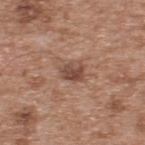workup — total-body-photography surveillance lesion; no biopsy | image — ~15 mm crop, total-body skin-cancer survey | automated lesion analysis — a border-irregularity rating of about 2.5/10; a classifier nevus-likeness of about 65/100 and a detector confidence of about 100 out of 100 that the crop contains a lesion | tile lighting — white-light | patient — male, aged around 65 | site — the upper back.